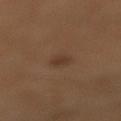Imaged with cross-polarized lighting.
Automated tile analysis of the lesion measured an average lesion color of about L≈38 a*≈17 b*≈26 (CIELAB) and roughly 6 lightness units darker than nearby skin. It also reported a border-irregularity rating of about 1.5/10.
A female subject roughly 55 years of age.
The lesion is on the right lower leg.
A region of skin cropped from a whole-body photographic capture, roughly 15 mm wide.